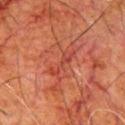Impression: Part of a total-body skin-imaging series; this lesion was reviewed on a skin check and was not flagged for biopsy. Context: Cropped from a total-body skin-imaging series; the visible field is about 15 mm. Longest diameter approximately 4 mm. A male subject about 80 years old. An algorithmic analysis of the crop reported a footprint of about 4 mm², an outline eccentricity of about 0.95 (0 = round, 1 = elongated), and two-axis asymmetry of about 0.5. And it measured a border-irregularity rating of about 7/10, internal color variation of about 1 on a 0–10 scale, and peripheral color asymmetry of about 0. On the chest.